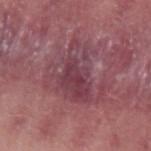Impression:
No biopsy was performed on this lesion — it was imaged during a full skin examination and was not determined to be concerning.
Acquisition and patient details:
The tile uses white-light illumination. Cropped from a total-body skin-imaging series; the visible field is about 15 mm. A male patient, about 30 years old. The lesion is located on the left upper arm. Automated image analysis of the tile measured an area of roughly 24 mm² and an outline eccentricity of about 0.65 (0 = round, 1 = elongated). The analysis additionally found a classifier nevus-likeness of about 0/100 and lesion-presence confidence of about 90/100. The recorded lesion diameter is about 7.5 mm.